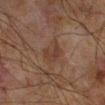The lesion was tiled from a total-body skin photograph and was not biopsied.
A lesion tile, about 15 mm wide, cut from a 3D total-body photograph.
The lesion is on the right lower leg.
A male patient aged 68 to 72.
Approximately 3.5 mm at its widest.